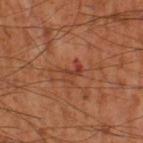Impression: Captured during whole-body skin photography for melanoma surveillance; the lesion was not biopsied. Acquisition and patient details: About 2.5 mm across. A region of skin cropped from a whole-body photographic capture, roughly 15 mm wide. A male patient aged 53–57. Located on the left thigh. Imaged with cross-polarized lighting.